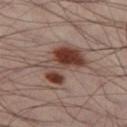Imaged during a routine full-body skin examination; the lesion was not biopsied and no histopathology is available. Approximately 5.5 mm at its widest. Cropped from a total-body skin-imaging series; the visible field is about 15 mm. A male subject aged 38–42. Located on the left thigh. Captured under cross-polarized illumination.